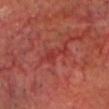{"biopsy_status": "not biopsied; imaged during a skin examination", "automated_metrics": {"area_mm2_approx": 4.0, "eccentricity": 0.9, "vs_skin_darker_L": 6.0, "border_irregularity_0_10": 5.5, "color_variation_0_10": 2.0, "peripheral_color_asymmetry": 0.5}, "lesion_size": {"long_diameter_mm_approx": 3.5}, "site": "head or neck", "image": {"source": "total-body photography crop", "field_of_view_mm": 15}, "patient": {"sex": "male", "age_approx": 65}}